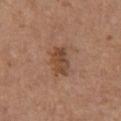Part of a total-body skin-imaging series; this lesion was reviewed on a skin check and was not flagged for biopsy. A female subject aged approximately 65. On the chest. Approximately 3.5 mm at its widest. A 15 mm close-up extracted from a 3D total-body photography capture.